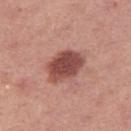| feature | finding |
|---|---|
| follow-up | no biopsy performed (imaged during a skin exam) |
| patient | female, about 40 years old |
| site | the right thigh |
| acquisition | total-body-photography crop, ~15 mm field of view |
| size | ~5 mm (longest diameter) |
| illumination | white-light |